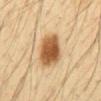Impression: The lesion was tiled from a total-body skin photograph and was not biopsied. Clinical summary: Located on the abdomen. A 15 mm close-up tile from a total-body photography series done for melanoma screening. Measured at roughly 4 mm in maximum diameter. Captured under cross-polarized illumination. A male subject, aged around 35.